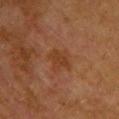biopsy status — total-body-photography surveillance lesion; no biopsy | lighting — cross-polarized | image — total-body-photography crop, ~15 mm field of view | anatomic site — the upper back | lesion diameter — about 3 mm | image-analysis metrics — an eccentricity of roughly 0.7 and a shape-asymmetry score of about 0.25 (0 = symmetric); a border-irregularity index near 3/10, a color-variation rating of about 1.5/10, and radial color variation of about 0.5; a classifier nevus-likeness of about 0/100 and lesion-presence confidence of about 100/100 | subject — female, approximately 55 years of age.